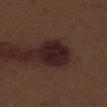Impression:
This lesion was catalogued during total-body skin photography and was not selected for biopsy.
Context:
Measured at roughly 4.5 mm in maximum diameter. The lesion is located on the right thigh. The total-body-photography lesion software estimated a lesion area of about 12 mm², an outline eccentricity of about 0.6 (0 = round, 1 = elongated), and a shape-asymmetry score of about 0.1 (0 = symmetric). The software also gave a lesion color around L≈20 a*≈17 b*≈15 in CIELAB, about 10 CIELAB-L* units darker than the surrounding skin, and a lesion-to-skin contrast of about 13 (normalized; higher = more distinct). And it measured a border-irregularity index near 1.5/10, internal color variation of about 2.5 on a 0–10 scale, and peripheral color asymmetry of about 1. A 15 mm close-up extracted from a 3D total-body photography capture. A male subject in their 70s.